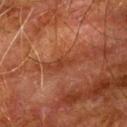Captured during whole-body skin photography for melanoma surveillance; the lesion was not biopsied. From the left upper arm. A region of skin cropped from a whole-body photographic capture, roughly 15 mm wide. A male subject, roughly 80 years of age.